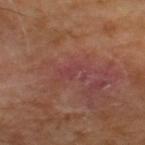Findings:
- follow-up · imaged on a skin check; not biopsied
- image · 15 mm crop, total-body photography
- lesion size · ~3.5 mm (longest diameter)
- illumination · cross-polarized illumination
- subject · male, about 65 years old
- automated lesion analysis · a lesion–skin lightness drop of about 4; border irregularity of about 6.5 on a 0–10 scale, internal color variation of about 1 on a 0–10 scale, and a peripheral color-asymmetry measure near 0; a nevus-likeness score of about 0/100 and lesion-presence confidence of about 95/100